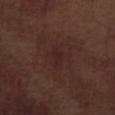<record>
<biopsy_status>not biopsied; imaged during a skin examination</biopsy_status>
<automated_metrics>
  <border_irregularity_0_10>3.0</border_irregularity_0_10>
  <color_variation_0_10>1.0</color_variation_0_10>
  <nevus_likeness_0_100>10</nevus_likeness_0_100>
  <lesion_detection_confidence_0_100>100</lesion_detection_confidence_0_100>
</automated_metrics>
<patient>
  <sex>male</sex>
  <age_approx>70</age_approx>
</patient>
<site>left lower leg</site>
<lighting>white-light</lighting>
<image>
  <source>total-body photography crop</source>
  <field_of_view_mm>15</field_of_view_mm>
</image>
<lesion_size>
  <long_diameter_mm_approx>3.0</long_diameter_mm_approx>
</lesion_size>
</record>